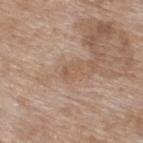Notes:
- follow-up — no biopsy performed (imaged during a skin exam)
- site — the upper back
- subject — female, roughly 75 years of age
- acquisition — ~15 mm tile from a whole-body skin photo